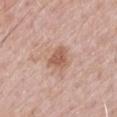Clinical impression:
Recorded during total-body skin imaging; not selected for excision or biopsy.
Background:
A male subject aged 63 to 67. Measured at roughly 3 mm in maximum diameter. A 15 mm close-up extracted from a 3D total-body photography capture. Captured under white-light illumination. The lesion is located on the arm.